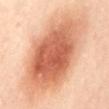| key | value |
|---|---|
| notes | imaged on a skin check; not biopsied |
| image | total-body-photography crop, ~15 mm field of view |
| lesion diameter | ~13 mm (longest diameter) |
| location | the back |
| patient | female, aged 38 to 42 |
| image-analysis metrics | an outline eccentricity of about 0.85 (0 = round, 1 = elongated) and a symmetry-axis asymmetry near 0.15; a lesion color around L≈64 a*≈26 b*≈36 in CIELAB, roughly 16 lightness units darker than nearby skin, and a lesion-to-skin contrast of about 9.5 (normalized; higher = more distinct); a nevus-likeness score of about 100/100 and a lesion-detection confidence of about 100/100 |
| tile lighting | cross-polarized |